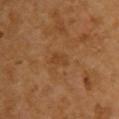Clinical impression: Imaged during a routine full-body skin examination; the lesion was not biopsied and no histopathology is available. Context: Imaged with cross-polarized lighting. The lesion is located on the upper back. A close-up tile cropped from a whole-body skin photograph, about 15 mm across. Measured at roughly 2.5 mm in maximum diameter. A female patient aged around 55.